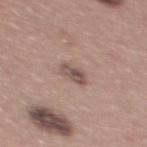No biopsy was performed on this lesion — it was imaged during a full skin examination and was not determined to be concerning. The lesion is on the mid back. A roughly 15 mm field-of-view crop from a total-body skin photograph. A male patient approximately 40 years of age. Imaged with white-light lighting. The lesion's longest dimension is about 3 mm.